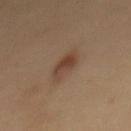Assessment:
The lesion was photographed on a routine skin check and not biopsied; there is no pathology result.
Acquisition and patient details:
The patient is a female about 50 years old. Approximately 4 mm at its widest. A lesion tile, about 15 mm wide, cut from a 3D total-body photograph.